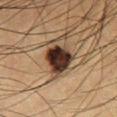Notes:
- follow-up · catalogued during a skin exam; not biopsied